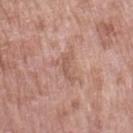• biopsy status · catalogued during a skin exam; not biopsied
• imaging modality · ~15 mm crop, total-body skin-cancer survey
• site · the arm
• diameter · ≈4 mm
• lighting · white-light illumination
• subject · female, aged 68–72